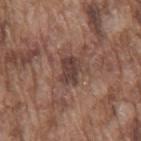Assessment: Imaged during a routine full-body skin examination; the lesion was not biopsied and no histopathology is available. Context: A male subject, roughly 75 years of age. A 15 mm crop from a total-body photograph taken for skin-cancer surveillance. The lesion is on the mid back.